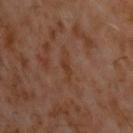Captured during whole-body skin photography for melanoma surveillance; the lesion was not biopsied.
Cropped from a whole-body photographic skin survey; the tile spans about 15 mm.
The lesion is located on the back.
Captured under cross-polarized illumination.
The recorded lesion diameter is about 3.5 mm.
Automated image analysis of the tile measured a border-irregularity index near 5/10.
A male subject roughly 60 years of age.